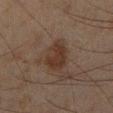Captured during whole-body skin photography for melanoma surveillance; the lesion was not biopsied. The lesion's longest dimension is about 4.5 mm. An algorithmic analysis of the crop reported an average lesion color of about L≈27 a*≈13 b*≈21 (CIELAB) and a lesion-to-skin contrast of about 7.5 (normalized; higher = more distinct). And it measured a border-irregularity rating of about 2.5/10, internal color variation of about 2.5 on a 0–10 scale, and radial color variation of about 1. The software also gave a nevus-likeness score of about 35/100 and a lesion-detection confidence of about 100/100. Cropped from a total-body skin-imaging series; the visible field is about 15 mm. Imaged with cross-polarized lighting. The lesion is on the right lower leg. The patient is a male aged 43 to 47.